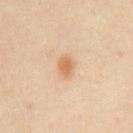  biopsy_status: not biopsied; imaged during a skin examination
  site: front of the torso
  automated_metrics:
    area_mm2_approx: 4.0
    eccentricity: 0.75
    shape_asymmetry: 0.15
    color_variation_0_10: 2.5
    nevus_likeness_0_100: 95
    lesion_detection_confidence_0_100: 100
  lesion_size:
    long_diameter_mm_approx: 3.0
  image:
    source: total-body photography crop
    field_of_view_mm: 15
  lighting: cross-polarized
  patient:
    sex: male
    age_approx: 45